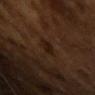Impression: This lesion was catalogued during total-body skin photography and was not selected for biopsy. Clinical summary: A 15 mm close-up tile from a total-body photography series done for melanoma screening. A male patient about 65 years old.